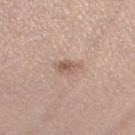Q: Is there a histopathology result?
A: no biopsy performed (imaged during a skin exam)
Q: How was this image acquired?
A: 15 mm crop, total-body photography
Q: Lesion size?
A: ≈3 mm
Q: Illumination type?
A: white-light illumination
Q: Where on the body is the lesion?
A: the left thigh
Q: Who is the patient?
A: female, aged 28 to 32